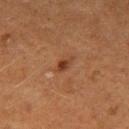Acquisition and patient details: The lesion is located on the right thigh. Captured under cross-polarized illumination. A region of skin cropped from a whole-body photographic capture, roughly 15 mm wide. The subject is a female approximately 50 years of age. Automated image analysis of the tile measured a lesion area of about 3 mm² and a shape eccentricity near 0.85. And it measured an average lesion color of about L≈33 a*≈20 b*≈28 (CIELAB) and roughly 8 lightness units darker than nearby skin. It also reported internal color variation of about 2.5 on a 0–10 scale.